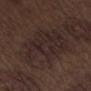follow-up = no biopsy performed (imaged during a skin exam)
location = the abdomen
patient = male, aged 68 to 72
image-analysis metrics = a lesion area of about 27 mm², a shape eccentricity near 0.4, and two-axis asymmetry of about 0.3; a border-irregularity rating of about 5.5/10 and peripheral color asymmetry of about 1; a nevus-likeness score of about 0/100 and lesion-presence confidence of about 75/100
illumination = white-light
lesion diameter = about 7.5 mm
image = 15 mm crop, total-body photography